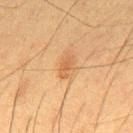Q: Was this lesion biopsied?
A: no biopsy performed (imaged during a skin exam)
Q: Who is the patient?
A: male, approximately 35 years of age
Q: Where on the body is the lesion?
A: the mid back
Q: What is the imaging modality?
A: 15 mm crop, total-body photography